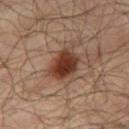<tbp_lesion>
  <biopsy_status>not biopsied; imaged during a skin examination</biopsy_status>
  <lighting>cross-polarized</lighting>
  <patient>
    <sex>male</sex>
    <age_approx>65</age_approx>
  </patient>
  <automated_metrics>
    <cielab_L>35</cielab_L>
    <cielab_a>21</cielab_a>
    <cielab_b>27</cielab_b>
  </automated_metrics>
  <site>right thigh</site>
  <lesion_size>
    <long_diameter_mm_approx>4.0</long_diameter_mm_approx>
  </lesion_size>
  <image>
    <source>total-body photography crop</source>
    <field_of_view_mm>15</field_of_view_mm>
  </image>
</tbp_lesion>